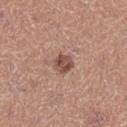Captured during whole-body skin photography for melanoma surveillance; the lesion was not biopsied.
A 15 mm crop from a total-body photograph taken for skin-cancer surveillance.
The patient is a female aged 38 to 42.
On the right lower leg.
An algorithmic analysis of the crop reported a lesion color around L≈49 a*≈22 b*≈25 in CIELAB, roughly 12 lightness units darker than nearby skin, and a normalized border contrast of about 8.5. It also reported a border-irregularity index near 2/10, internal color variation of about 4 on a 0–10 scale, and radial color variation of about 1.5. It also reported an automated nevus-likeness rating near 50 out of 100 and a detector confidence of about 100 out of 100 that the crop contains a lesion.
Longest diameter approximately 2.5 mm.